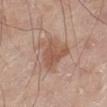<case>
<biopsy_status>not biopsied; imaged during a skin examination</biopsy_status>
<lighting>white-light</lighting>
<site>leg</site>
<automated_metrics>
  <area_mm2_approx>12.0</area_mm2_approx>
  <eccentricity>0.4</eccentricity>
  <shape_asymmetry>0.35</shape_asymmetry>
  <border_irregularity_0_10>4.0</border_irregularity_0_10>
  <color_variation_0_10>3.0</color_variation_0_10>
  <peripheral_color_asymmetry>1.0</peripheral_color_asymmetry>
  <nevus_likeness_0_100>45</nevus_likeness_0_100>
  <lesion_detection_confidence_0_100>100</lesion_detection_confidence_0_100>
</automated_metrics>
<patient>
  <sex>male</sex>
  <age_approx>80</age_approx>
</patient>
<image>
  <source>total-body photography crop</source>
  <field_of_view_mm>15</field_of_view_mm>
</image>
<lesion_size>
  <long_diameter_mm_approx>4.5</long_diameter_mm_approx>
</lesion_size>
</case>